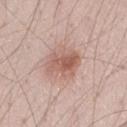The lesion was photographed on a routine skin check and not biopsied; there is no pathology result. This image is a 15 mm lesion crop taken from a total-body photograph. The lesion-visualizer software estimated a shape eccentricity near 0.65. It also reported a lesion color around L≈58 a*≈21 b*≈26 in CIELAB, about 11 CIELAB-L* units darker than the surrounding skin, and a normalized lesion–skin contrast near 7.5. And it measured a nevus-likeness score of about 65/100 and a detector confidence of about 100 out of 100 that the crop contains a lesion. Captured under white-light illumination. A male patient, aged approximately 40. The lesion is located on the right thigh. The recorded lesion diameter is about 4 mm.